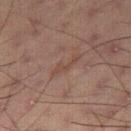Assessment: This lesion was catalogued during total-body skin photography and was not selected for biopsy.